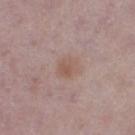This lesion was catalogued during total-body skin photography and was not selected for biopsy. Imaged with white-light lighting. On the right thigh. The subject is a female approximately 30 years of age. Longest diameter approximately 3 mm. A roughly 15 mm field-of-view crop from a total-body skin photograph.